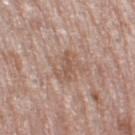Assessment:
The lesion was tiled from a total-body skin photograph and was not biopsied.
Context:
A female subject in their mid- to late 60s. A lesion tile, about 15 mm wide, cut from a 3D total-body photograph. On the leg. Automated tile analysis of the lesion measured a shape eccentricity near 0.75 and a symmetry-axis asymmetry near 0.5. And it measured a mean CIELAB color near L≈55 a*≈18 b*≈27, a lesion–skin lightness drop of about 8, and a normalized lesion–skin contrast near 5.5. The software also gave a border-irregularity index near 6.5/10, a within-lesion color-variation index near 2.5/10, and peripheral color asymmetry of about 1. It also reported a nevus-likeness score of about 0/100 and lesion-presence confidence of about 100/100. The lesion's longest dimension is about 3.5 mm. Imaged with white-light lighting.